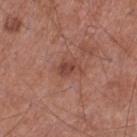follow-up: catalogued during a skin exam; not biopsied | lighting: white-light | image: 15 mm crop, total-body photography | lesion diameter: ~2.5 mm (longest diameter) | subject: male, approximately 55 years of age | anatomic site: the left thigh.